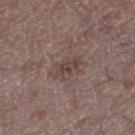<tbp_lesion>
<biopsy_status>not biopsied; imaged during a skin examination</biopsy_status>
<patient>
  <sex>male</sex>
  <age_approx>50</age_approx>
</patient>
<site>left thigh</site>
<image>
  <source>total-body photography crop</source>
  <field_of_view_mm>15</field_of_view_mm>
</image>
<lighting>white-light</lighting>
<lesion_size>
  <long_diameter_mm_approx>3.0</long_diameter_mm_approx>
</lesion_size>
</tbp_lesion>